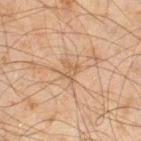acquisition — total-body-photography crop, ~15 mm field of view | lesion diameter — ~2.5 mm (longest diameter) | subject — male, in their mid- to late 40s | site — the right thigh | lighting — cross-polarized illumination.